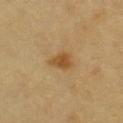  biopsy_status: not biopsied; imaged during a skin examination
  site: front of the torso
  patient:
    sex: male
    age_approx: 60
  image:
    source: total-body photography crop
    field_of_view_mm: 15